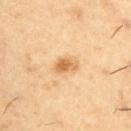Imaged during a routine full-body skin examination; the lesion was not biopsied and no histopathology is available. The lesion is located on the right upper arm. A 15 mm crop from a total-body photograph taken for skin-cancer surveillance. The patient is a male aged around 55.